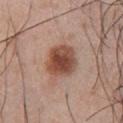Captured during whole-body skin photography for melanoma surveillance; the lesion was not biopsied. A male patient, aged 53–57. The lesion is located on the chest. A lesion tile, about 15 mm wide, cut from a 3D total-body photograph. The tile uses white-light illumination. The total-body-photography lesion software estimated a classifier nevus-likeness of about 100/100 and a detector confidence of about 100 out of 100 that the crop contains a lesion.